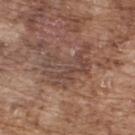No biopsy was performed on this lesion — it was imaged during a full skin examination and was not determined to be concerning.
Cropped from a whole-body photographic skin survey; the tile spans about 15 mm.
The patient is a male aged 73 to 77.
The lesion is located on the upper back.
About 6 mm across.
The tile uses white-light illumination.